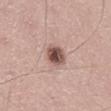Q: Is there a histopathology result?
A: imaged on a skin check; not biopsied
Q: Lesion size?
A: ~2.5 mm (longest diameter)
Q: Lesion location?
A: the leg
Q: How was this image acquired?
A: ~15 mm crop, total-body skin-cancer survey
Q: Patient demographics?
A: male, aged approximately 60
Q: How was the tile lit?
A: white-light illumination
Q: What did automated image analysis measure?
A: a footprint of about 6 mm² and two-axis asymmetry of about 0.15; a mean CIELAB color near L≈51 a*≈20 b*≈23, roughly 16 lightness units darker than nearby skin, and a normalized border contrast of about 11